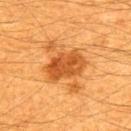Clinical impression:
Imaged during a routine full-body skin examination; the lesion was not biopsied and no histopathology is available.
Background:
A male patient about 60 years old. Located on the upper back. A close-up tile cropped from a whole-body skin photograph, about 15 mm across. The lesion's longest dimension is about 5 mm. The tile uses cross-polarized illumination.